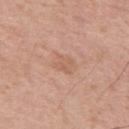Imaged during a routine full-body skin examination; the lesion was not biopsied and no histopathology is available. A region of skin cropped from a whole-body photographic capture, roughly 15 mm wide. The recorded lesion diameter is about 2.5 mm. The total-body-photography lesion software estimated a border-irregularity index near 3/10, a color-variation rating of about 2/10, and radial color variation of about 0.5. Imaged with white-light lighting. A male subject, roughly 65 years of age. The lesion is on the upper back.